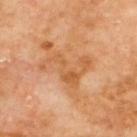Clinical impression:
Imaged during a routine full-body skin examination; the lesion was not biopsied and no histopathology is available.
Image and clinical context:
A male patient, aged around 70. Cropped from a total-body skin-imaging series; the visible field is about 15 mm. On the upper back. Automated tile analysis of the lesion measured an outline eccentricity of about 0.8 (0 = round, 1 = elongated) and a symmetry-axis asymmetry near 0.75. It also reported roughly 8 lightness units darker than nearby skin and a normalized border contrast of about 6. And it measured a border-irregularity index near 10/10 and a within-lesion color-variation index near 2.5/10. The analysis additionally found a nevus-likeness score of about 0/100 and a lesion-detection confidence of about 100/100. Imaged with cross-polarized lighting.